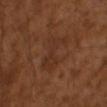follow-up = imaged on a skin check; not biopsied
patient = male, aged around 65
diameter = about 5.5 mm
image source = ~15 mm tile from a whole-body skin photo